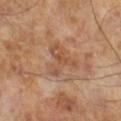Assessment: Recorded during total-body skin imaging; not selected for excision or biopsy. Image and clinical context: Longest diameter approximately 4.5 mm. Captured under cross-polarized illumination. A region of skin cropped from a whole-body photographic capture, roughly 15 mm wide. The subject is a male approximately 65 years of age.